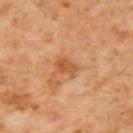Impression:
Part of a total-body skin-imaging series; this lesion was reviewed on a skin check and was not flagged for biopsy.
Acquisition and patient details:
Cropped from a whole-body photographic skin survey; the tile spans about 15 mm. A male subject, aged approximately 60. The lesion is located on the upper back.